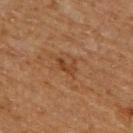| key | value |
|---|---|
| site | the upper back |
| image source | ~15 mm tile from a whole-body skin photo |
| lighting | cross-polarized |
| patient | male, about 65 years old |